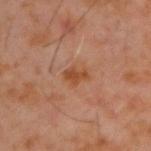notes = total-body-photography surveillance lesion; no biopsy
imaging modality = 15 mm crop, total-body photography
subject = male, aged approximately 60
body site = the upper back